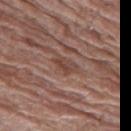<lesion>
<biopsy_status>not biopsied; imaged during a skin examination</biopsy_status>
<patient>
  <sex>female</sex>
  <age_approx>80</age_approx>
</patient>
<image>
  <source>total-body photography crop</source>
  <field_of_view_mm>15</field_of_view_mm>
</image>
<site>left thigh</site>
<lesion_size>
  <long_diameter_mm_approx>3.0</long_diameter_mm_approx>
</lesion_size>
<lighting>white-light</lighting>
</lesion>